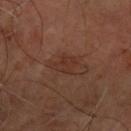Case summary:
* follow-up: total-body-photography surveillance lesion; no biopsy
* automated lesion analysis: a lesion–skin lightness drop of about 5 and a normalized border contrast of about 5.5; an automated nevus-likeness rating near 0 out of 100
* size: ~2.5 mm (longest diameter)
* tile lighting: cross-polarized illumination
* image: ~15 mm tile from a whole-body skin photo
* subject: aged approximately 65
* body site: the right forearm